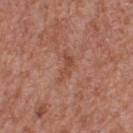The lesion was photographed on a routine skin check and not biopsied; there is no pathology result. A 15 mm close-up tile from a total-body photography series done for melanoma screening. The lesion is on the back. The patient is a male aged around 65.